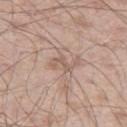Impression:
Recorded during total-body skin imaging; not selected for excision or biopsy.
Image and clinical context:
A region of skin cropped from a whole-body photographic capture, roughly 15 mm wide. Approximately 3.5 mm at its widest. An algorithmic analysis of the crop reported a lesion area of about 4.5 mm² and a symmetry-axis asymmetry near 0.5. And it measured a mean CIELAB color near L≈58 a*≈17 b*≈25. It also reported a border-irregularity index near 6/10, a color-variation rating of about 1.5/10, and peripheral color asymmetry of about 0.5. The patient is a male about 55 years old. Captured under white-light illumination. The lesion is on the left thigh.